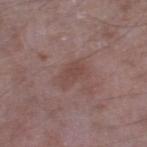Impression:
The lesion was photographed on a routine skin check and not biopsied; there is no pathology result.
Acquisition and patient details:
A male patient aged 48–52. The recorded lesion diameter is about 3.5 mm. A 15 mm close-up tile from a total-body photography series done for melanoma screening. Captured under white-light illumination. The total-body-photography lesion software estimated roughly 6 lightness units darker than nearby skin and a normalized lesion–skin contrast near 5. On the right lower leg.